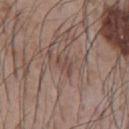Assessment:
This lesion was catalogued during total-body skin photography and was not selected for biopsy.
Context:
The subject is a male aged 73–77. This is a white-light tile. Located on the chest. About 2.5 mm across. Cropped from a whole-body photographic skin survey; the tile spans about 15 mm.